Located on the head or neck. A 15 mm close-up extracted from a 3D total-body photography capture. The patient is a male aged 58 to 62 — 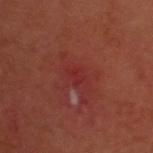Conclusion: On biopsy, histopathology showed a malignancy: squamous cell carcinoma in situ.